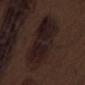Q: Is there a histopathology result?
A: imaged on a skin check; not biopsied
Q: How large is the lesion?
A: ≈10 mm
Q: Who is the patient?
A: male, in their 70s
Q: What kind of image is this?
A: 15 mm crop, total-body photography
Q: Lesion location?
A: the right thigh
Q: Automated lesion metrics?
A: an area of roughly 34 mm²; a border-irregularity rating of about 2.5/10, internal color variation of about 5.5 on a 0–10 scale, and a peripheral color-asymmetry measure near 2